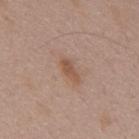Recorded during total-body skin imaging; not selected for excision or biopsy. Automated image analysis of the tile measured a lesion area of about 3.5 mm² and a shape eccentricity near 0.9. It also reported border irregularity of about 3.5 on a 0–10 scale and a peripheral color-asymmetry measure near 0. It also reported a nevus-likeness score of about 20/100 and a detector confidence of about 100 out of 100 that the crop contains a lesion. A roughly 15 mm field-of-view crop from a total-body skin photograph. On the mid back. This is a white-light tile. The subject is a male approximately 50 years of age.